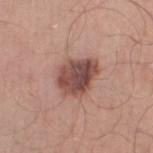Recorded during total-body skin imaging; not selected for excision or biopsy. Imaged with white-light lighting. On the left thigh. The patient is a male in their 50s. Automated image analysis of the tile measured an average lesion color of about L≈48 a*≈21 b*≈24 (CIELAB). Cropped from a total-body skin-imaging series; the visible field is about 15 mm. Approximately 5 mm at its widest.